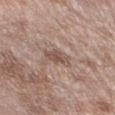| feature | finding |
|---|---|
| biopsy status | catalogued during a skin exam; not biopsied |
| lesion size | about 3 mm |
| anatomic site | the right forearm |
| imaging modality | total-body-photography crop, ~15 mm field of view |
| TBP lesion metrics | a footprint of about 5 mm², an outline eccentricity of about 0.8 (0 = round, 1 = elongated), and two-axis asymmetry of about 0.3; a border-irregularity index near 3.5/10 and internal color variation of about 2 on a 0–10 scale; a classifier nevus-likeness of about 0/100 and lesion-presence confidence of about 100/100 |
| subject | male, approximately 70 years of age |
| illumination | white-light illumination |